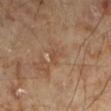Q: Is there a histopathology result?
A: catalogued during a skin exam; not biopsied
Q: Who is the patient?
A: male, in their 70s
Q: What is the lesion's diameter?
A: about 2.5 mm
Q: How was the tile lit?
A: cross-polarized illumination
Q: What did automated image analysis measure?
A: an average lesion color of about L≈47 a*≈18 b*≈30 (CIELAB) and a lesion–skin lightness drop of about 6; a nevus-likeness score of about 0/100 and a lesion-detection confidence of about 95/100
Q: What is the anatomic site?
A: the leg
Q: What kind of image is this?
A: total-body-photography crop, ~15 mm field of view